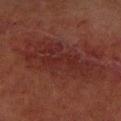Part of a total-body skin-imaging series; this lesion was reviewed on a skin check and was not flagged for biopsy.
A close-up tile cropped from a whole-body skin photograph, about 15 mm across.
The lesion is located on the left lower leg.
A male patient approximately 70 years of age.